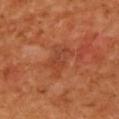* follow-up: catalogued during a skin exam; not biopsied
* location: the upper back
* image source: total-body-photography crop, ~15 mm field of view
* lesion size: ≈4 mm
* automated lesion analysis: an area of roughly 9 mm² and a symmetry-axis asymmetry near 0.3
* patient: female, in their 40s